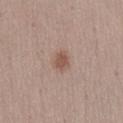Imaged during a routine full-body skin examination; the lesion was not biopsied and no histopathology is available. A 15 mm crop from a total-body photograph taken for skin-cancer surveillance. From the abdomen. A female subject, roughly 30 years of age. This is a white-light tile. The recorded lesion diameter is about 2.5 mm.